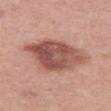Captured during whole-body skin photography for melanoma surveillance; the lesion was not biopsied.
Captured under white-light illumination.
The lesion is located on the left thigh.
A 15 mm close-up extracted from a 3D total-body photography capture.
A female subject about 40 years old.